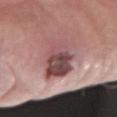No biopsy was performed on this lesion — it was imaged during a full skin examination and was not determined to be concerning. A 15 mm close-up extracted from a 3D total-body photography capture. Approximately 7 mm at its widest. Imaged with white-light lighting. The lesion-visualizer software estimated a shape eccentricity near 0.9 and a symmetry-axis asymmetry near 0.2. The analysis additionally found border irregularity of about 3.5 on a 0–10 scale, a within-lesion color-variation index near 9.5/10, and a peripheral color-asymmetry measure near 4. And it measured lesion-presence confidence of about 85/100. A male patient aged 53–57.